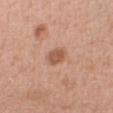{"biopsy_status": "not biopsied; imaged during a skin examination", "site": "right forearm", "patient": {"sex": "male", "age_approx": 50}, "image": {"source": "total-body photography crop", "field_of_view_mm": 15}}